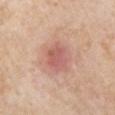biopsy_status: not biopsied; imaged during a skin examination
patient:
  sex: male
  age_approx: 85+
image:
  source: total-body photography crop
  field_of_view_mm: 15
lesion_size:
  long_diameter_mm_approx: 3.5
automated_metrics:
  area_mm2_approx: 9.5
  eccentricity: 0.5
site: chest
lighting: white-light